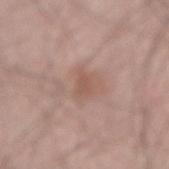Clinical impression: The lesion was photographed on a routine skin check and not biopsied; there is no pathology result. Image and clinical context: The lesion is located on the lower back. A male patient, in their mid-60s. A 15 mm crop from a total-body photograph taken for skin-cancer surveillance.